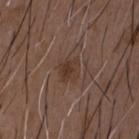Q: Is there a histopathology result?
A: catalogued during a skin exam; not biopsied
Q: How was this image acquired?
A: ~15 mm crop, total-body skin-cancer survey
Q: Who is the patient?
A: male, about 50 years old
Q: Illumination type?
A: white-light illumination
Q: Automated lesion metrics?
A: an average lesion color of about L≈36 a*≈17 b*≈24 (CIELAB) and a normalized border contrast of about 6.5; radial color variation of about 1
Q: Where on the body is the lesion?
A: the chest
Q: How large is the lesion?
A: ~2.5 mm (longest diameter)